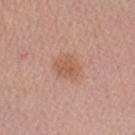notes = imaged on a skin check; not biopsied | acquisition = 15 mm crop, total-body photography | lighting = white-light | anatomic site = the arm | subject = female, aged approximately 65.